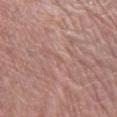Impression: The lesion was photographed on a routine skin check and not biopsied; there is no pathology result. Context: The lesion is located on the right lower leg. Automated image analysis of the tile measured an area of roughly 1 mm², a shape eccentricity near 0.85, and two-axis asymmetry of about 0.45. And it measured a lesion color around L≈57 a*≈20 b*≈26 in CIELAB, a lesion–skin lightness drop of about 4, and a normalized border contrast of about 3. A close-up tile cropped from a whole-body skin photograph, about 15 mm across. Imaged with white-light lighting. The recorded lesion diameter is about 1.5 mm. A female patient aged around 60.